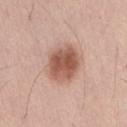notes = imaged on a skin check; not biopsied
anatomic site = the lower back
patient = male, aged 53 to 57
image = total-body-photography crop, ~15 mm field of view